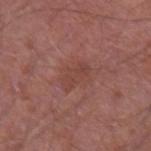Clinical impression: This lesion was catalogued during total-body skin photography and was not selected for biopsy. Context: From the left forearm. A 15 mm crop from a total-body photograph taken for skin-cancer surveillance. A male subject, aged 48 to 52.